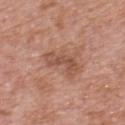biopsy status: imaged on a skin check; not biopsied
site: the chest
patient: male, roughly 70 years of age
imaging modality: ~15 mm tile from a whole-body skin photo